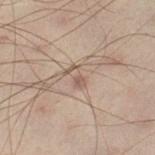• notes: catalogued during a skin exam; not biopsied
• tile lighting: cross-polarized illumination
• size: ~2.5 mm (longest diameter)
• subject: male, approximately 50 years of age
• imaging modality: ~15 mm tile from a whole-body skin photo
• site: the leg